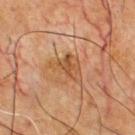No biopsy was performed on this lesion — it was imaged during a full skin examination and was not determined to be concerning. Imaged with cross-polarized lighting. A male patient aged 63 to 67. This image is a 15 mm lesion crop taken from a total-body photograph. From the chest. An algorithmic analysis of the crop reported a footprint of about 8.5 mm² and two-axis asymmetry of about 0.5. The software also gave about 7 CIELAB-L* units darker than the surrounding skin and a normalized border contrast of about 6.5. The software also gave a border-irregularity index near 5/10, a color-variation rating of about 3/10, and peripheral color asymmetry of about 1.